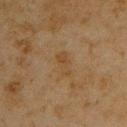tile lighting: cross-polarized illumination; diameter: ~3 mm (longest diameter); acquisition: 15 mm crop, total-body photography; patient: male, approximately 45 years of age; anatomic site: the upper back; automated lesion analysis: a footprint of about 4 mm², an outline eccentricity of about 0.9 (0 = round, 1 = elongated), and two-axis asymmetry of about 0.4.